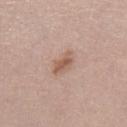{"image": {"source": "total-body photography crop", "field_of_view_mm": 15}, "site": "left lower leg", "patient": {"sex": "female", "age_approx": 40}}